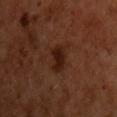Recorded during total-body skin imaging; not selected for excision or biopsy.
An algorithmic analysis of the crop reported an area of roughly 6.5 mm² and an outline eccentricity of about 0.75 (0 = round, 1 = elongated). The analysis additionally found a normalized lesion–skin contrast near 9. The software also gave a border-irregularity index near 2.5/10 and peripheral color asymmetry of about 0.5. The software also gave a lesion-detection confidence of about 100/100.
A region of skin cropped from a whole-body photographic capture, roughly 15 mm wide.
The recorded lesion diameter is about 3.5 mm.
A male patient, approximately 50 years of age.
The lesion is located on the chest.